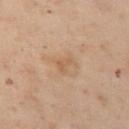Part of a total-body skin-imaging series; this lesion was reviewed on a skin check and was not flagged for biopsy. The lesion is located on the chest. A region of skin cropped from a whole-body photographic capture, roughly 15 mm wide. The patient is a female aged around 55.